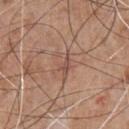follow-up — no biopsy performed (imaged during a skin exam) | subject — male, about 65 years old | image — total-body-photography crop, ~15 mm field of view | location — the chest.